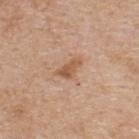Part of a total-body skin-imaging series; this lesion was reviewed on a skin check and was not flagged for biopsy. A male patient about 60 years old. Cropped from a whole-body photographic skin survey; the tile spans about 15 mm. This is a white-light tile. Automated image analysis of the tile measured an area of roughly 4 mm² and an eccentricity of roughly 0.85. The analysis additionally found a mean CIELAB color near L≈56 a*≈21 b*≈33, roughly 10 lightness units darker than nearby skin, and a normalized lesion–skin contrast near 7. The analysis additionally found an automated nevus-likeness rating near 10 out of 100. The lesion's longest dimension is about 3 mm. From the upper back.